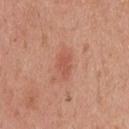No biopsy was performed on this lesion — it was imaged during a full skin examination and was not determined to be concerning. Located on the head or neck. Captured under white-light illumination. This image is a 15 mm lesion crop taken from a total-body photograph. A male patient in their mid- to late 20s. The lesion's longest dimension is about 3.5 mm.